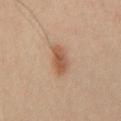Imaged during a routine full-body skin examination; the lesion was not biopsied and no histopathology is available. A region of skin cropped from a whole-body photographic capture, roughly 15 mm wide. A male subject, aged around 40. Located on the front of the torso. An algorithmic analysis of the crop reported a lesion area of about 6 mm². The tile uses cross-polarized illumination.